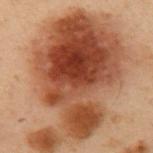workup = catalogued during a skin exam; not biopsied | lesion diameter = ~16 mm (longest diameter) | image = total-body-photography crop, ~15 mm field of view | patient = male, in their mid-50s | location = the left upper arm.